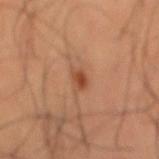  biopsy_status: not biopsied; imaged during a skin examination
  lighting: cross-polarized
  image:
    source: total-body photography crop
    field_of_view_mm: 15
  site: mid back
  patient:
    sex: male
    age_approx: 65